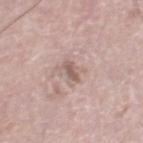Q: Is there a histopathology result?
A: no biopsy performed (imaged during a skin exam)
Q: Lesion location?
A: the right thigh
Q: What is the lesion's diameter?
A: ~3 mm (longest diameter)
Q: What is the imaging modality?
A: total-body-photography crop, ~15 mm field of view
Q: What are the patient's age and sex?
A: male, roughly 75 years of age